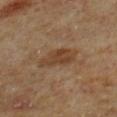Assessment:
The lesion was tiled from a total-body skin photograph and was not biopsied.
Acquisition and patient details:
Located on the left lower leg. The patient is a male approximately 60 years of age. Imaged with cross-polarized lighting. A lesion tile, about 15 mm wide, cut from a 3D total-body photograph.